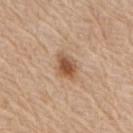Recorded during total-body skin imaging; not selected for excision or biopsy.
Automated tile analysis of the lesion measured an average lesion color of about L≈54 a*≈20 b*≈33 (CIELAB), about 13 CIELAB-L* units darker than the surrounding skin, and a lesion-to-skin contrast of about 9 (normalized; higher = more distinct). It also reported border irregularity of about 2 on a 0–10 scale and a color-variation rating of about 5/10. The software also gave an automated nevus-likeness rating near 95 out of 100.
The lesion is located on the left arm.
The subject is a female approximately 65 years of age.
This is a white-light tile.
Longest diameter approximately 3.5 mm.
A roughly 15 mm field-of-view crop from a total-body skin photograph.